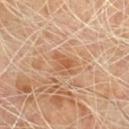notes — total-body-photography surveillance lesion; no biopsy | image-analysis metrics — an area of roughly 4.5 mm²; an average lesion color of about L≈56 a*≈22 b*≈36 (CIELAB), a lesion–skin lightness drop of about 8, and a lesion-to-skin contrast of about 6.5 (normalized; higher = more distinct); a border-irregularity rating of about 3/10 and peripheral color asymmetry of about 1 | imaging modality — ~15 mm crop, total-body skin-cancer survey | body site — the front of the torso | patient — male, approximately 70 years of age.